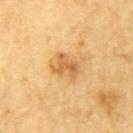The lesion is located on the left upper arm. This image is a 15 mm lesion crop taken from a total-body photograph. The tile uses cross-polarized illumination. A male subject, aged around 85 or older.